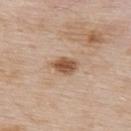  biopsy_status: not biopsied; imaged during a skin examination
  patient:
    sex: male
    age_approx: 55
  image:
    source: total-body photography crop
    field_of_view_mm: 15
  automated_metrics:
    eccentricity: 0.75
    shape_asymmetry: 0.25
    cielab_L: 53
    cielab_a: 20
    cielab_b: 32
    vs_skin_darker_L: 14.0
    vs_skin_contrast_norm: 9.5
    border_irregularity_0_10: 2.0
    color_variation_0_10: 3.5
    peripheral_color_asymmetry: 1.0
  lesion_size:
    long_diameter_mm_approx: 3.0
  site: upper back